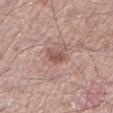A 15 mm crop from a total-body photograph taken for skin-cancer surveillance.
The recorded lesion diameter is about 2.5 mm.
A male patient aged 58 to 62.
The total-body-photography lesion software estimated an area of roughly 4 mm², an outline eccentricity of about 0.7 (0 = round, 1 = elongated), and two-axis asymmetry of about 0.35. The software also gave border irregularity of about 3.5 on a 0–10 scale, a color-variation rating of about 1.5/10, and peripheral color asymmetry of about 0.5. The analysis additionally found a classifier nevus-likeness of about 35/100 and a detector confidence of about 100 out of 100 that the crop contains a lesion.
This is a white-light tile.
The lesion is located on the left lower leg.